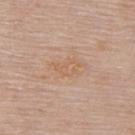follow-up: no biopsy performed (imaged during a skin exam); subject: female, aged approximately 65; imaging modality: ~15 mm tile from a whole-body skin photo; location: the upper back.